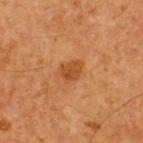No biopsy was performed on this lesion — it was imaged during a full skin examination and was not determined to be concerning.
From the upper back.
This is a cross-polarized tile.
A region of skin cropped from a whole-body photographic capture, roughly 15 mm wide.
The subject is a male aged 63–67.
Measured at roughly 3 mm in maximum diameter.
The lesion-visualizer software estimated a lesion area of about 4.5 mm², an eccentricity of roughly 0.65, and two-axis asymmetry of about 0.25. The software also gave a border-irregularity rating of about 2/10, a color-variation rating of about 2/10, and peripheral color asymmetry of about 0.5.